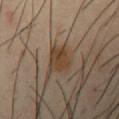Clinical impression: Imaged during a routine full-body skin examination; the lesion was not biopsied and no histopathology is available. Acquisition and patient details: The lesion is located on the left upper arm. A close-up tile cropped from a whole-body skin photograph, about 15 mm across. A male patient, aged around 40. The lesion-visualizer software estimated an area of roughly 5.5 mm², a shape eccentricity near 0.7, and a symmetry-axis asymmetry near 0.25. The software also gave internal color variation of about 3 on a 0–10 scale and radial color variation of about 1. The tile uses cross-polarized illumination.